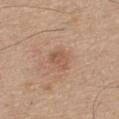Case summary:
* follow-up — total-body-photography surveillance lesion; no biopsy
* automated lesion analysis — a border-irregularity rating of about 2.5/10, a color-variation rating of about 2.5/10, and peripheral color asymmetry of about 1; a nevus-likeness score of about 10/100 and a lesion-detection confidence of about 100/100
* patient — male, about 65 years old
* lesion size — about 3 mm
* imaging modality — 15 mm crop, total-body photography
* lighting — white-light illumination
* site — the upper back